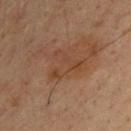The lesion was photographed on a routine skin check and not biopsied; there is no pathology result. The lesion's longest dimension is about 4.5 mm. This image is a 15 mm lesion crop taken from a total-body photograph. The tile uses cross-polarized illumination. On the back. A male patient aged around 35.